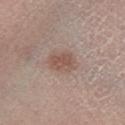| field | value |
|---|---|
| follow-up | no biopsy performed (imaged during a skin exam) |
| image-analysis metrics | a nevus-likeness score of about 85/100 and a lesion-detection confidence of about 100/100 |
| tile lighting | white-light |
| patient | female, approximately 30 years of age |
| image | ~15 mm tile from a whole-body skin photo |
| location | the left lower leg |